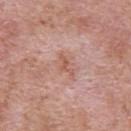biopsy status: total-body-photography surveillance lesion; no biopsy
subject: male, aged around 50
site: the upper back
automated metrics: an outline eccentricity of about 0.9 (0 = round, 1 = elongated) and a symmetry-axis asymmetry near 0.65; a lesion color around L≈56 a*≈24 b*≈28 in CIELAB, roughly 8 lightness units darker than nearby skin, and a normalized lesion–skin contrast near 6.5; a border-irregularity index near 7/10, a color-variation rating of about 0/10, and radial color variation of about 0; an automated nevus-likeness rating near 0 out of 100
image source: ~15 mm crop, total-body skin-cancer survey
diameter: ~3 mm (longest diameter)